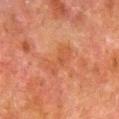A male patient, aged approximately 80. On the right lower leg. A lesion tile, about 15 mm wide, cut from a 3D total-body photograph. The recorded lesion diameter is about 4.5 mm. Imaged with cross-polarized lighting.